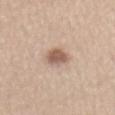| key | value |
|---|---|
| biopsy status | catalogued during a skin exam; not biopsied |
| anatomic site | the front of the torso |
| patient | male, aged 33 to 37 |
| acquisition | 15 mm crop, total-body photography |